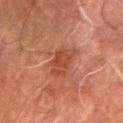Q: Was this lesion biopsied?
A: total-body-photography surveillance lesion; no biopsy
Q: Patient demographics?
A: male, approximately 80 years of age
Q: What is the anatomic site?
A: the right forearm
Q: What is the imaging modality?
A: 15 mm crop, total-body photography